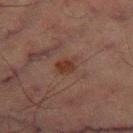The lesion was tiled from a total-body skin photograph and was not biopsied. A 15 mm close-up extracted from a 3D total-body photography capture. A male patient, in their mid-60s. The lesion is located on the left thigh. Measured at roughly 2.5 mm in maximum diameter.